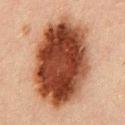Assessment: Recorded during total-body skin imaging; not selected for excision or biopsy. Context: An algorithmic analysis of the crop reported a symmetry-axis asymmetry near 0.1. The software also gave a border-irregularity index near 2/10 and radial color variation of about 3. On the chest. A male subject aged 48 to 52. A 15 mm close-up extracted from a 3D total-body photography capture.